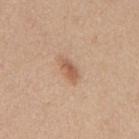notes = imaged on a skin check; not biopsied